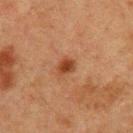Assessment:
The lesion was photographed on a routine skin check and not biopsied; there is no pathology result.
Image and clinical context:
A male subject approximately 60 years of age. About 2.5 mm across. From the upper back. Imaged with cross-polarized lighting. A 15 mm crop from a total-body photograph taken for skin-cancer surveillance.